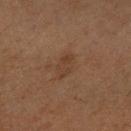No biopsy was performed on this lesion — it was imaged during a full skin examination and was not determined to be concerning. A region of skin cropped from a whole-body photographic capture, roughly 15 mm wide. A male subject, aged 58 to 62. The lesion is on the right upper arm.